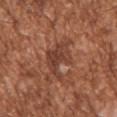Impression:
Recorded during total-body skin imaging; not selected for excision or biopsy.
Clinical summary:
Measured at roughly 4 mm in maximum diameter. The lesion is on the right upper arm. The subject is a male aged approximately 45. A lesion tile, about 15 mm wide, cut from a 3D total-body photograph.